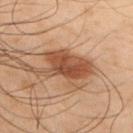No biopsy was performed on this lesion — it was imaged during a full skin examination and was not determined to be concerning. A region of skin cropped from a whole-body photographic capture, roughly 15 mm wide. An algorithmic analysis of the crop reported a symmetry-axis asymmetry near 0.3. A male subject, roughly 50 years of age. The lesion is on the right upper arm.